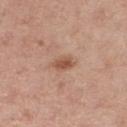Recorded during total-body skin imaging; not selected for excision or biopsy. A female patient aged approximately 45. Approximately 2.5 mm at its widest. The tile uses white-light illumination. From the right thigh. Cropped from a total-body skin-imaging series; the visible field is about 15 mm.